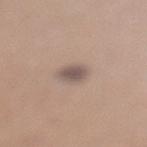Assessment: Part of a total-body skin-imaging series; this lesion was reviewed on a skin check and was not flagged for biopsy. Clinical summary: The lesion is located on the left lower leg. Captured under white-light illumination. Automated tile analysis of the lesion measured a lesion area of about 5 mm² and a shape eccentricity near 0.65. A female patient, aged around 45. A roughly 15 mm field-of-view crop from a total-body skin photograph.